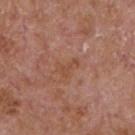The lesion was photographed on a routine skin check and not biopsied; there is no pathology result. The lesion's longest dimension is about 3 mm. A 15 mm close-up tile from a total-body photography series done for melanoma screening. The lesion-visualizer software estimated border irregularity of about 6.5 on a 0–10 scale and internal color variation of about 0 on a 0–10 scale. The software also gave a classifier nevus-likeness of about 0/100 and a lesion-detection confidence of about 100/100. From the chest. A male patient aged 63 to 67.